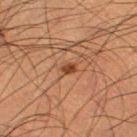Findings:
* workup · total-body-photography surveillance lesion; no biopsy
* location · the right thigh
* automated metrics · roughly 10 lightness units darker than nearby skin and a lesion-to-skin contrast of about 9 (normalized; higher = more distinct); border irregularity of about 2 on a 0–10 scale
* acquisition · ~15 mm crop, total-body skin-cancer survey
* subject · male, aged approximately 50
* lighting · cross-polarized illumination
* lesion diameter · ≈2 mm